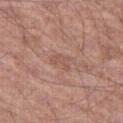Clinical impression:
Imaged during a routine full-body skin examination; the lesion was not biopsied and no histopathology is available.
Context:
A 15 mm close-up extracted from a 3D total-body photography capture. The lesion is located on the leg. Measured at roughly 2.5 mm in maximum diameter. This is a white-light tile. A male subject in their mid-60s.